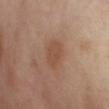Findings:
* notes — imaged on a skin check; not biopsied
* imaging modality — 15 mm crop, total-body photography
* patient — female, in their mid- to late 50s
* lesion diameter — ≈3.5 mm
* tile lighting — cross-polarized
* location — the abdomen